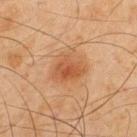The lesion was photographed on a routine skin check and not biopsied; there is no pathology result.
This image is a 15 mm lesion crop taken from a total-body photograph.
A male subject aged 43 to 47.
The total-body-photography lesion software estimated a lesion area of about 12 mm² and an outline eccentricity of about 0.55 (0 = round, 1 = elongated). And it measured an automated nevus-likeness rating near 90 out of 100 and a lesion-detection confidence of about 100/100.
On the upper back.
Captured under cross-polarized illumination.
Measured at roughly 4 mm in maximum diameter.